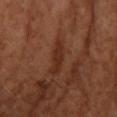  biopsy_status: not biopsied; imaged during a skin examination
  automated_metrics:
    area_mm2_approx: 3.0
    eccentricity: 0.95
    shape_asymmetry: 0.4
  patient:
    sex: female
    age_approx: 65
  lesion_size:
    long_diameter_mm_approx: 3.5
  site: right forearm
  lighting: cross-polarized
  image:
    source: total-body photography crop
    field_of_view_mm: 15